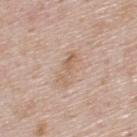{"biopsy_status": "not biopsied; imaged during a skin examination", "lesion_size": {"long_diameter_mm_approx": 4.0}, "lighting": "white-light", "automated_metrics": {"area_mm2_approx": 4.5, "eccentricity": 0.95, "cielab_L": 61, "cielab_a": 17, "cielab_b": 30, "vs_skin_contrast_norm": 5.5}, "patient": {"sex": "male", "age_approx": 45}, "site": "upper back", "image": {"source": "total-body photography crop", "field_of_view_mm": 15}}